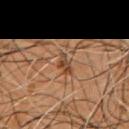| field | value |
|---|---|
| biopsy status | total-body-photography surveillance lesion; no biopsy |
| tile lighting | cross-polarized |
| size | about 3 mm |
| subject | male, aged 53 to 57 |
| body site | the chest |
| automated lesion analysis | an eccentricity of roughly 0.85 and a shape-asymmetry score of about 0.4 (0 = symmetric); a classifier nevus-likeness of about 0/100 and a detector confidence of about 75 out of 100 that the crop contains a lesion |
| image | ~15 mm crop, total-body skin-cancer survey |